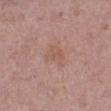notes: total-body-photography surveillance lesion; no biopsy
body site: the right lower leg
lesion size: ≈3 mm
patient: female, roughly 40 years of age
tile lighting: white-light illumination
image-analysis metrics: a footprint of about 5 mm², a shape eccentricity near 0.7, and two-axis asymmetry of about 0.35; a lesion color around L≈55 a*≈21 b*≈26 in CIELAB, roughly 6 lightness units darker than nearby skin, and a normalized border contrast of about 4.5; lesion-presence confidence of about 100/100
acquisition: ~15 mm tile from a whole-body skin photo